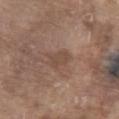Clinical impression:
The lesion was photographed on a routine skin check and not biopsied; there is no pathology result.
Image and clinical context:
A male subject aged around 80. The total-body-photography lesion software estimated a classifier nevus-likeness of about 0/100 and lesion-presence confidence of about 100/100. The lesion is on the abdomen. Cropped from a whole-body photographic skin survey; the tile spans about 15 mm. The tile uses white-light illumination.